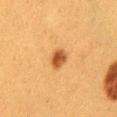The lesion was tiled from a total-body skin photograph and was not biopsied.
Automated image analysis of the tile measured a shape eccentricity near 0.65. The software also gave about 13 CIELAB-L* units darker than the surrounding skin and a normalized border contrast of about 9.5.
The subject is a female approximately 40 years of age.
This image is a 15 mm lesion crop taken from a total-body photograph.
The lesion is located on the mid back.